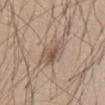biopsy status = catalogued during a skin exam; not biopsied | tile lighting = white-light illumination | patient = male, about 45 years old | lesion size = about 4 mm | image source = ~15 mm crop, total-body skin-cancer survey | body site = the front of the torso.